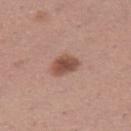Clinical impression: Recorded during total-body skin imaging; not selected for excision or biopsy.